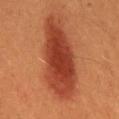<record>
<biopsy_status>not biopsied; imaged during a skin examination</biopsy_status>
<automated_metrics>
  <area_mm2_approx>34.0</area_mm2_approx>
  <eccentricity>0.95</eccentricity>
  <shape_asymmetry>0.2</shape_asymmetry>
</automated_metrics>
<image>
  <source>total-body photography crop</source>
  <field_of_view_mm>15</field_of_view_mm>
</image>
<site>mid back</site>
<lighting>cross-polarized</lighting>
<patient>
  <sex>male</sex>
  <age_approx>60</age_approx>
</patient>
<lesion_size>
  <long_diameter_mm_approx>11.5</long_diameter_mm_approx>
</lesion_size>
</record>